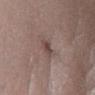Clinical impression:
No biopsy was performed on this lesion — it was imaged during a full skin examination and was not determined to be concerning.
Context:
The recorded lesion diameter is about 2.5 mm. A male subject, aged 43 to 47. From the leg. A 15 mm close-up tile from a total-body photography series done for melanoma screening.